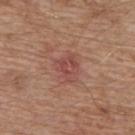Captured during whole-body skin photography for melanoma surveillance; the lesion was not biopsied. Approximately 3 mm at its widest. A 15 mm close-up extracted from a 3D total-body photography capture. The lesion is located on the back. A male subject, roughly 65 years of age. Imaged with white-light lighting.